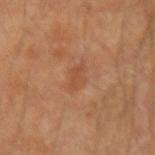Q: Was a biopsy performed?
A: imaged on a skin check; not biopsied
Q: Illumination type?
A: cross-polarized illumination
Q: Patient demographics?
A: male, about 40 years old
Q: What is the anatomic site?
A: the right forearm
Q: What is the imaging modality?
A: 15 mm crop, total-body photography
Q: What did automated image analysis measure?
A: an average lesion color of about L≈50 a*≈24 b*≈35 (CIELAB), roughly 6 lightness units darker than nearby skin, and a lesion-to-skin contrast of about 4.5 (normalized; higher = more distinct); a border-irregularity rating of about 2.5/10 and radial color variation of about 0.5; an automated nevus-likeness rating near 0 out of 100 and a lesion-detection confidence of about 100/100
Q: Lesion size?
A: ≈3.5 mm